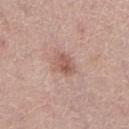<lesion>
<biopsy_status>not biopsied; imaged during a skin examination</biopsy_status>
<site>right lower leg</site>
<automated_metrics>
  <area_mm2_approx>5.0</area_mm2_approx>
  <eccentricity>0.75</eccentricity>
  <shape_asymmetry>0.2</shape_asymmetry>
  <vs_skin_darker_L>10.0</vs_skin_darker_L>
  <vs_skin_contrast_norm>6.5</vs_skin_contrast_norm>
  <border_irregularity_0_10>1.5</border_irregularity_0_10>
  <color_variation_0_10>4.0</color_variation_0_10>
  <peripheral_color_asymmetry>1.5</peripheral_color_asymmetry>
  <lesion_detection_confidence_0_100>100</lesion_detection_confidence_0_100>
</automated_metrics>
<lighting>white-light</lighting>
<patient>
  <sex>female</sex>
  <age_approx>60</age_approx>
</patient>
<image>
  <source>total-body photography crop</source>
  <field_of_view_mm>15</field_of_view_mm>
</image>
</lesion>